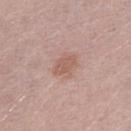The subject is a male aged 28 to 32. The tile uses white-light illumination. Approximately 3.5 mm at its widest. An algorithmic analysis of the crop reported a footprint of about 6.5 mm², a shape eccentricity near 0.65, and a shape-asymmetry score of about 0.2 (0 = symmetric). The software also gave a mean CIELAB color near L≈58 a*≈20 b*≈26, about 8 CIELAB-L* units darker than the surrounding skin, and a normalized lesion–skin contrast near 6. It also reported an automated nevus-likeness rating near 25 out of 100 and lesion-presence confidence of about 100/100. A region of skin cropped from a whole-body photographic capture, roughly 15 mm wide.